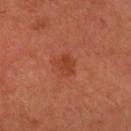lesion diameter = ≈2.5 mm | subject = male, approximately 60 years of age | acquisition = ~15 mm tile from a whole-body skin photo | site = the right forearm.